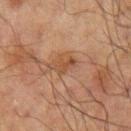biopsy_status: not biopsied; imaged during a skin examination
image:
  source: total-body photography crop
  field_of_view_mm: 15
lesion_size:
  long_diameter_mm_approx: 2.5
site: left upper arm
lighting: cross-polarized
patient:
  sex: male
  age_approx: 70